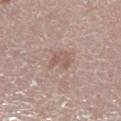Background: The tile uses white-light illumination. A region of skin cropped from a whole-body photographic capture, roughly 15 mm wide. Longest diameter approximately 2.5 mm. The lesion is on the left lower leg. The patient is a female about 65 years old.